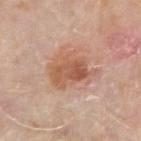workup = total-body-photography surveillance lesion; no biopsy
body site = the right forearm
lighting = white-light
image = total-body-photography crop, ~15 mm field of view
subject = male, aged approximately 75
size = ~5 mm (longest diameter)